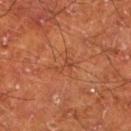The lesion was photographed on a routine skin check and not biopsied; there is no pathology result. A male subject, aged approximately 65. The lesion is on the left lower leg. The recorded lesion diameter is about 2.5 mm. The total-body-photography lesion software estimated a footprint of about 2.5 mm² and a shape-asymmetry score of about 0.5 (0 = symmetric). It also reported a mean CIELAB color near L≈44 a*≈27 b*≈34. And it measured a border-irregularity rating of about 6/10 and peripheral color asymmetry of about 0. And it measured a nevus-likeness score of about 0/100. A 15 mm crop from a total-body photograph taken for skin-cancer surveillance. This is a cross-polarized tile.